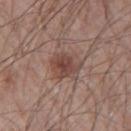Clinical impression:
The lesion was photographed on a routine skin check and not biopsied; there is no pathology result.
Context:
The patient is a male aged 63 to 67. Imaged with white-light lighting. The lesion is on the right upper arm. This image is a 15 mm lesion crop taken from a total-body photograph.